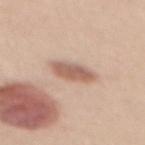The lesion was photographed on a routine skin check and not biopsied; there is no pathology result. On the mid back. A female patient roughly 45 years of age. Measured at roughly 4.5 mm in maximum diameter. An algorithmic analysis of the crop reported a footprint of about 7.5 mm², a shape eccentricity near 0.85, and two-axis asymmetry of about 0.2. The software also gave a peripheral color-asymmetry measure near 1. The software also gave an automated nevus-likeness rating near 80 out of 100 and a lesion-detection confidence of about 100/100. This is a white-light tile. This image is a 15 mm lesion crop taken from a total-body photograph.